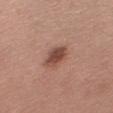Notes:
- workup: imaged on a skin check; not biopsied